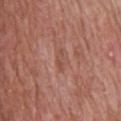{"biopsy_status": "not biopsied; imaged during a skin examination"}